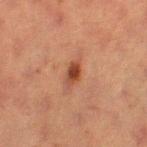{
  "biopsy_status": "not biopsied; imaged during a skin examination",
  "automated_metrics": {
    "nevus_likeness_0_100": 90,
    "lesion_detection_confidence_0_100": 100
  },
  "site": "left thigh",
  "patient": {
    "sex": "female",
    "age_approx": 55
  },
  "lighting": "cross-polarized",
  "lesion_size": {
    "long_diameter_mm_approx": 3.0
  },
  "image": {
    "source": "total-body photography crop",
    "field_of_view_mm": 15
  }
}